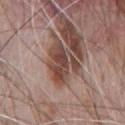The lesion was tiled from a total-body skin photograph and was not biopsied. Cropped from a total-body skin-imaging series; the visible field is about 15 mm. Automated tile analysis of the lesion measured an eccentricity of roughly 0.7 and a shape-asymmetry score of about 0.5 (0 = symmetric). The analysis additionally found a color-variation rating of about 5/10 and a peripheral color-asymmetry measure near 1.5. On the chest. This is a white-light tile. A male subject, aged approximately 70.